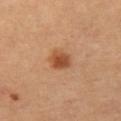Clinical summary: This is a cross-polarized tile. A 15 mm crop from a total-body photograph taken for skin-cancer surveillance. The patient is a female aged 58–62. The lesion is on the left thigh.